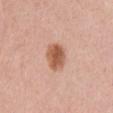notes: total-body-photography surveillance lesion; no biopsy | image: total-body-photography crop, ~15 mm field of view | lesion size: ≈3.5 mm | site: the chest | patient: female, roughly 35 years of age.